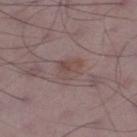No biopsy was performed on this lesion — it was imaged during a full skin examination and was not determined to be concerning.
The subject is a male about 50 years old.
Located on the left thigh.
A 15 mm close-up tile from a total-body photography series done for melanoma screening.